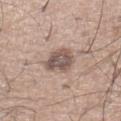{
  "site": "left lower leg",
  "image": {
    "source": "total-body photography crop",
    "field_of_view_mm": 15
  },
  "patient": {
    "sex": "male",
    "age_approx": 30
  },
  "lighting": "white-light",
  "lesion_size": {
    "long_diameter_mm_approx": 3.5
  }
}